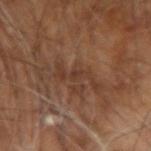site: the right arm
acquisition: 15 mm crop, total-body photography
lighting: cross-polarized
lesion size: about 5 mm
patient: male, about 60 years old